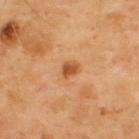Imaged during a routine full-body skin examination; the lesion was not biopsied and no histopathology is available. From the upper back. The subject is a male about 70 years old. A region of skin cropped from a whole-body photographic capture, roughly 15 mm wide. An algorithmic analysis of the crop reported an average lesion color of about L≈52 a*≈26 b*≈41 (CIELAB), about 12 CIELAB-L* units darker than the surrounding skin, and a normalized lesion–skin contrast near 8.5. The software also gave a detector confidence of about 100 out of 100 that the crop contains a lesion. The tile uses cross-polarized illumination.